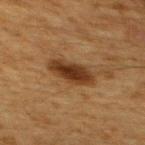Part of a total-body skin-imaging series; this lesion was reviewed on a skin check and was not flagged for biopsy. A male subject approximately 60 years of age. A 15 mm close-up tile from a total-body photography series done for melanoma screening. About 4.5 mm across. Automated tile analysis of the lesion measured a footprint of about 10 mm², a shape eccentricity near 0.85, and two-axis asymmetry of about 0.2. It also reported border irregularity of about 2.5 on a 0–10 scale and a peripheral color-asymmetry measure near 1. And it measured a classifier nevus-likeness of about 90/100 and a lesion-detection confidence of about 100/100. The lesion is on the upper back. Imaged with cross-polarized lighting.